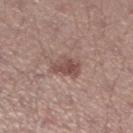The lesion was tiled from a total-body skin photograph and was not biopsied.